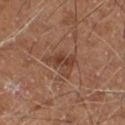Clinical impression: Part of a total-body skin-imaging series; this lesion was reviewed on a skin check and was not flagged for biopsy. Acquisition and patient details: About 3.5 mm across. This is a cross-polarized tile. A male subject, aged 58–62. Cropped from a total-body skin-imaging series; the visible field is about 15 mm. The lesion is located on the right thigh.